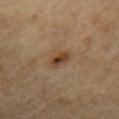Recorded during total-body skin imaging; not selected for excision or biopsy.
A male patient aged 68 to 72.
A 15 mm close-up tile from a total-body photography series done for melanoma screening.
The tile uses cross-polarized illumination.
Automated tile analysis of the lesion measured a lesion area of about 4.5 mm². The software also gave a border-irregularity index near 2.5/10, a color-variation rating of about 6/10, and radial color variation of about 2.
Located on the left upper arm.